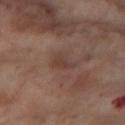Clinical impression:
The lesion was photographed on a routine skin check and not biopsied; there is no pathology result.
Background:
A region of skin cropped from a whole-body photographic capture, roughly 15 mm wide. A female subject roughly 55 years of age. The lesion is located on the right thigh.